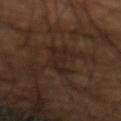Q: Was a biopsy performed?
A: imaged on a skin check; not biopsied
Q: Lesion size?
A: ≈5 mm
Q: What lighting was used for the tile?
A: cross-polarized illumination
Q: Lesion location?
A: the arm
Q: Who is the patient?
A: male, about 60 years old
Q: How was this image acquired?
A: ~15 mm crop, total-body skin-cancer survey
Q: What did automated image analysis measure?
A: a footprint of about 7 mm² and a shape-asymmetry score of about 0.4 (0 = symmetric); border irregularity of about 6 on a 0–10 scale, a within-lesion color-variation index near 1.5/10, and peripheral color asymmetry of about 0.5; an automated nevus-likeness rating near 20 out of 100